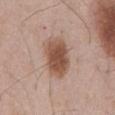Q: Was a biopsy performed?
A: no biopsy performed (imaged during a skin exam)
Q: Automated lesion metrics?
A: an average lesion color of about L≈51 a*≈20 b*≈28 (CIELAB) and a lesion-to-skin contrast of about 10 (normalized; higher = more distinct); border irregularity of about 1 on a 0–10 scale, a color-variation rating of about 3.5/10, and radial color variation of about 1; lesion-presence confidence of about 100/100
Q: Patient demographics?
A: male, in their mid-50s
Q: How was the tile lit?
A: white-light
Q: What is the imaging modality?
A: total-body-photography crop, ~15 mm field of view
Q: Lesion location?
A: the abdomen
Q: Lesion size?
A: ~5 mm (longest diameter)